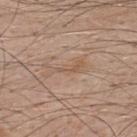Q: Is there a histopathology result?
A: total-body-photography surveillance lesion; no biopsy
Q: Lesion size?
A: about 5 mm
Q: Automated lesion metrics?
A: a border-irregularity index near 7.5/10, a within-lesion color-variation index near 0/10, and radial color variation of about 0
Q: Where on the body is the lesion?
A: the upper back
Q: What kind of image is this?
A: ~15 mm tile from a whole-body skin photo
Q: How was the tile lit?
A: white-light
Q: Patient demographics?
A: male, approximately 50 years of age